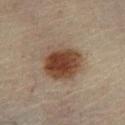| key | value |
|---|---|
| follow-up | no biopsy performed (imaged during a skin exam) |
| image source | ~15 mm tile from a whole-body skin photo |
| size | ≈5 mm |
| body site | the left lower leg |
| patient | male, aged approximately 45 |